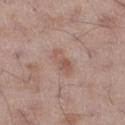Imaged during a routine full-body skin examination; the lesion was not biopsied and no histopathology is available. On the left thigh. Approximately 3.5 mm at its widest. A 15 mm crop from a total-body photograph taken for skin-cancer surveillance. A male patient, aged approximately 50.